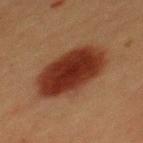<tbp_lesion>
  <biopsy_status>not biopsied; imaged during a skin examination</biopsy_status>
</tbp_lesion>